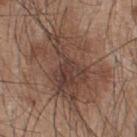imaging modality = 15 mm crop, total-body photography
location = the upper back
size = ≈9.5 mm
patient = male, aged approximately 45
lighting = white-light illumination